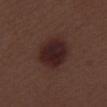Assessment: Part of a total-body skin-imaging series; this lesion was reviewed on a skin check and was not flagged for biopsy. Background: Imaged with white-light lighting. The total-body-photography lesion software estimated a symmetry-axis asymmetry near 0.1. The analysis additionally found a lesion color around L≈23 a*≈19 b*≈17 in CIELAB and a normalized lesion–skin contrast near 11. It also reported a border-irregularity index near 1/10, internal color variation of about 4 on a 0–10 scale, and peripheral color asymmetry of about 1. Located on the right thigh. The recorded lesion diameter is about 5 mm. A 15 mm crop from a total-body photograph taken for skin-cancer surveillance. The subject is a male roughly 70 years of age.